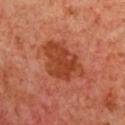workup = total-body-photography surveillance lesion; no biopsy
subject = female, roughly 40 years of age
lighting = cross-polarized
site = the chest
lesion size = ~6 mm (longest diameter)
image source = 15 mm crop, total-body photography